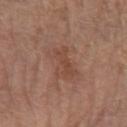location = the left forearm; lesion size = about 4 mm; image source = total-body-photography crop, ~15 mm field of view; patient = female, in their mid- to late 50s; illumination = white-light.